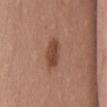tile lighting: white-light
acquisition: ~15 mm tile from a whole-body skin photo
automated lesion analysis: a lesion area of about 7.5 mm², an eccentricity of roughly 0.9, and two-axis asymmetry of about 0.15; a within-lesion color-variation index near 2.5/10 and peripheral color asymmetry of about 1
subject: female, aged around 50
body site: the abdomen